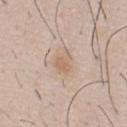| key | value |
|---|---|
| follow-up | catalogued during a skin exam; not biopsied |
| image-analysis metrics | a lesion area of about 4.5 mm² and an outline eccentricity of about 0.7 (0 = round, 1 = elongated); a lesion color around L≈63 a*≈16 b*≈30 in CIELAB and a lesion-to-skin contrast of about 6 (normalized; higher = more distinct); a border-irregularity rating of about 3/10, internal color variation of about 2 on a 0–10 scale, and a peripheral color-asymmetry measure near 0.5; a nevus-likeness score of about 40/100 and a lesion-detection confidence of about 100/100 |
| location | the front of the torso |
| lighting | white-light |
| size | ~3 mm (longest diameter) |
| patient | male, roughly 40 years of age |
| acquisition | 15 mm crop, total-body photography |